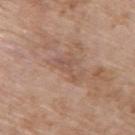Q: What kind of image is this?
A: ~15 mm crop, total-body skin-cancer survey
Q: Who is the patient?
A: female, in their mid- to late 70s
Q: What is the anatomic site?
A: the upper back
Q: What is the lesion's diameter?
A: about 3.5 mm
Q: What lighting was used for the tile?
A: white-light